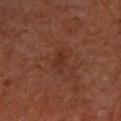This lesion was catalogued during total-body skin photography and was not selected for biopsy. Automated tile analysis of the lesion measured radial color variation of about 0.5. This is a cross-polarized tile. From the left forearm. A 15 mm crop from a total-body photograph taken for skin-cancer surveillance. A female subject, about 65 years old. The lesion's longest dimension is about 3.5 mm.